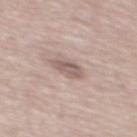Recorded during total-body skin imaging; not selected for excision or biopsy. A male patient, aged around 80. A lesion tile, about 15 mm wide, cut from a 3D total-body photograph. The lesion is located on the mid back.